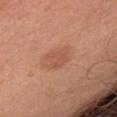From the left upper arm.
A lesion tile, about 15 mm wide, cut from a 3D total-body photograph.
A male subject, approximately 70 years of age.